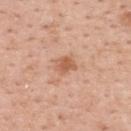Captured during whole-body skin photography for melanoma surveillance; the lesion was not biopsied.
The subject is a female about 50 years old.
A 15 mm close-up tile from a total-body photography series done for melanoma screening.
The tile uses white-light illumination.
Automated tile analysis of the lesion measured an outline eccentricity of about 0.65 (0 = round, 1 = elongated) and a shape-asymmetry score of about 0.25 (0 = symmetric). The analysis additionally found a lesion-detection confidence of about 100/100.
On the upper back.
Longest diameter approximately 2.5 mm.